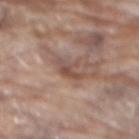A close-up tile cropped from a whole-body skin photograph, about 15 mm across.
A male patient, aged 78–82.
The lesion is on the mid back.